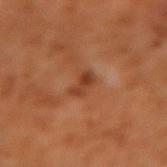Clinical impression: The lesion was tiled from a total-body skin photograph and was not biopsied. Acquisition and patient details: From the left forearm. The subject is a male aged 58 to 62. A 15 mm close-up extracted from a 3D total-body photography capture. The total-body-photography lesion software estimated an area of roughly 3.5 mm², an outline eccentricity of about 0.9 (0 = round, 1 = elongated), and two-axis asymmetry of about 0.35. The software also gave a normalized lesion–skin contrast near 7.5. The software also gave a border-irregularity rating of about 4/10, a color-variation rating of about 2/10, and radial color variation of about 0.